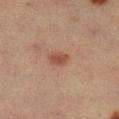Captured during whole-body skin photography for melanoma surveillance; the lesion was not biopsied. A 15 mm crop from a total-body photograph taken for skin-cancer surveillance. A female patient aged 53 to 57. The total-body-photography lesion software estimated a lesion–skin lightness drop of about 8 and a lesion-to-skin contrast of about 7 (normalized; higher = more distinct). The analysis additionally found border irregularity of about 2.5 on a 0–10 scale, a within-lesion color-variation index near 2/10, and a peripheral color-asymmetry measure near 0.5. The analysis additionally found a classifier nevus-likeness of about 90/100. Captured under cross-polarized illumination. The lesion is on the right lower leg.